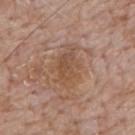Imaged during a routine full-body skin examination; the lesion was not biopsied and no histopathology is available.
The subject is a male aged around 60.
Measured at roughly 4.5 mm in maximum diameter.
On the mid back.
Captured under white-light illumination.
A lesion tile, about 15 mm wide, cut from a 3D total-body photograph.